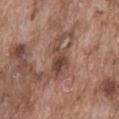notes: total-body-photography surveillance lesion; no biopsy
tile lighting: white-light
automated metrics: a footprint of about 7.5 mm²; a lesion color around L≈45 a*≈20 b*≈25 in CIELAB, a lesion–skin lightness drop of about 10, and a lesion-to-skin contrast of about 8 (normalized; higher = more distinct); border irregularity of about 6.5 on a 0–10 scale, a color-variation rating of about 3.5/10, and peripheral color asymmetry of about 1.5; an automated nevus-likeness rating near 0 out of 100 and a detector confidence of about 95 out of 100 that the crop contains a lesion
image: ~15 mm crop, total-body skin-cancer survey
size: ≈5.5 mm
subject: male, roughly 75 years of age
site: the front of the torso